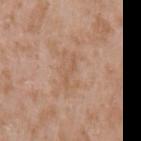Q: Was this lesion biopsied?
A: total-body-photography surveillance lesion; no biopsy
Q: Patient demographics?
A: male, about 50 years old
Q: What is the imaging modality?
A: ~15 mm crop, total-body skin-cancer survey
Q: Lesion location?
A: the left upper arm